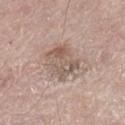follow-up = total-body-photography surveillance lesion; no biopsy
automated metrics = an area of roughly 11 mm², an eccentricity of roughly 0.75, and two-axis asymmetry of about 0.2; a border-irregularity index near 2.5/10, a color-variation rating of about 5.5/10, and a peripheral color-asymmetry measure near 1.5; an automated nevus-likeness rating near 10 out of 100 and a detector confidence of about 100 out of 100 that the crop contains a lesion
lighting = white-light illumination
subject = male, about 75 years old
lesion diameter = ~4.5 mm (longest diameter)
acquisition = 15 mm crop, total-body photography
anatomic site = the left thigh